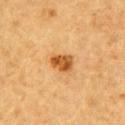follow-up: total-body-photography surveillance lesion; no biopsy | automated lesion analysis: a lesion area of about 5.5 mm² and a shape-asymmetry score of about 0.3 (0 = symmetric); roughly 13 lightness units darker than nearby skin and a normalized border contrast of about 9.5; an automated nevus-likeness rating near 95 out of 100 and a detector confidence of about 100 out of 100 that the crop contains a lesion | size: ≈3 mm | illumination: cross-polarized illumination | subject: male, roughly 85 years of age or older | body site: the right upper arm | acquisition: total-body-photography crop, ~15 mm field of view.